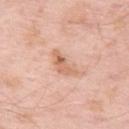workup: no biopsy performed (imaged during a skin exam) | location: the right thigh | size: ~4.5 mm (longest diameter) | illumination: white-light illumination | automated lesion analysis: a lesion area of about 6.5 mm², an outline eccentricity of about 0.9 (0 = round, 1 = elongated), and a symmetry-axis asymmetry near 0.4; a mean CIELAB color near L≈66 a*≈22 b*≈32, about 9 CIELAB-L* units darker than the surrounding skin, and a normalized lesion–skin contrast near 6.5; a border-irregularity rating of about 4.5/10 and a within-lesion color-variation index near 6.5/10; a classifier nevus-likeness of about 0/100 and a lesion-detection confidence of about 100/100 | image source: ~15 mm crop, total-body skin-cancer survey | subject: male, about 60 years old.